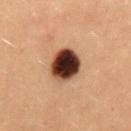Captured during whole-body skin photography for melanoma surveillance; the lesion was not biopsied. From the mid back. An algorithmic analysis of the crop reported a lesion color around L≈28 a*≈18 b*≈22 in CIELAB, about 23 CIELAB-L* units darker than the surrounding skin, and a normalized lesion–skin contrast near 20. And it measured a border-irregularity rating of about 1/10, a color-variation rating of about 7.5/10, and radial color variation of about 2. Cropped from a total-body skin-imaging series; the visible field is about 15 mm. The patient is a female aged 18 to 22. Measured at roughly 4 mm in maximum diameter. Captured under cross-polarized illumination.